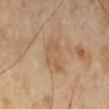Impression:
The lesion was photographed on a routine skin check and not biopsied; there is no pathology result.
Background:
The lesion is located on the right lower leg. Imaged with cross-polarized lighting. The recorded lesion diameter is about 4.5 mm. A female subject, about 70 years old. A 15 mm close-up tile from a total-body photography series done for melanoma screening. The total-body-photography lesion software estimated a border-irregularity index near 3/10 and a peripheral color-asymmetry measure near 1.